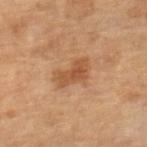<record>
<biopsy_status>not biopsied; imaged during a skin examination</biopsy_status>
<automated_metrics>
  <area_mm2_approx>7.5</area_mm2_approx>
  <eccentricity>0.85</eccentricity>
  <shape_asymmetry>0.3</shape_asymmetry>
  <cielab_L>50</cielab_L>
  <cielab_a>22</cielab_a>
  <cielab_b>35</cielab_b>
  <vs_skin_darker_L>9.0</vs_skin_darker_L>
  <vs_skin_contrast_norm>7.0</vs_skin_contrast_norm>
  <color_variation_0_10>3.5</color_variation_0_10>
  <peripheral_color_asymmetry>1.0</peripheral_color_asymmetry>
  <nevus_likeness_0_100>10</nevus_likeness_0_100>
  <lesion_detection_confidence_0_100>100</lesion_detection_confidence_0_100>
</automated_metrics>
<lighting>cross-polarized</lighting>
<site>right lower leg</site>
<image>
  <source>total-body photography crop</source>
  <field_of_view_mm>15</field_of_view_mm>
</image>
<patient>
  <sex>female</sex>
  <age_approx>70</age_approx>
</patient>
</record>